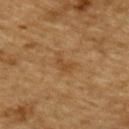No biopsy was performed on this lesion — it was imaged during a full skin examination and was not determined to be concerning.
Cropped from a total-body skin-imaging series; the visible field is about 15 mm.
The patient is a male aged 83 or over.
About 2.5 mm across.
On the upper back.
An algorithmic analysis of the crop reported a lesion area of about 3 mm², an eccentricity of roughly 0.85, and a symmetry-axis asymmetry near 0.5.